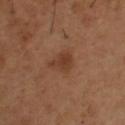Findings:
- workup: no biopsy performed (imaged during a skin exam)
- site: the upper back
- subject: male, aged around 55
- image: ~15 mm tile from a whole-body skin photo